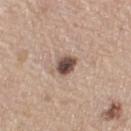Recorded during total-body skin imaging; not selected for excision or biopsy.
The total-body-photography lesion software estimated a symmetry-axis asymmetry near 0.25. And it measured a lesion color around L≈49 a*≈16 b*≈23 in CIELAB and roughly 17 lightness units darker than nearby skin.
The recorded lesion diameter is about 2.5 mm.
A lesion tile, about 15 mm wide, cut from a 3D total-body photograph.
The lesion is on the right thigh.
A female patient, aged approximately 70.
Imaged with white-light lighting.